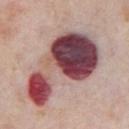Assessment:
Imaged during a routine full-body skin examination; the lesion was not biopsied and no histopathology is available.
Image and clinical context:
A 15 mm crop from a total-body photograph taken for skin-cancer surveillance. The lesion is on the chest. Imaged with white-light lighting. The total-body-photography lesion software estimated a footprint of about 42 mm², an outline eccentricity of about 0.9 (0 = round, 1 = elongated), and a symmetry-axis asymmetry near 0.5. The software also gave a lesion color around L≈45 a*≈26 b*≈17 in CIELAB and roughly 24 lightness units darker than nearby skin. The recorded lesion diameter is about 11 mm. A male patient about 75 years old.